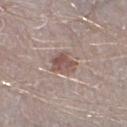<case>
<biopsy_status>not biopsied; imaged during a skin examination</biopsy_status>
<lighting>white-light</lighting>
<lesion_size>
  <long_diameter_mm_approx>3.0</long_diameter_mm_approx>
</lesion_size>
<patient>
  <sex>male</sex>
  <age_approx>40</age_approx>
</patient>
<image>
  <source>total-body photography crop</source>
  <field_of_view_mm>15</field_of_view_mm>
</image>
<site>left lower leg</site>
</case>